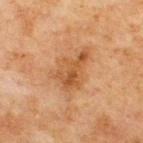| feature | finding |
|---|---|
| notes | no biopsy performed (imaged during a skin exam) |
| TBP lesion metrics | a border-irregularity rating of about 5.5/10 and a color-variation rating of about 5/10 |
| anatomic site | the back |
| image source | ~15 mm tile from a whole-body skin photo |
| patient | male, roughly 70 years of age |
| lesion size | ≈6 mm |
| illumination | cross-polarized |